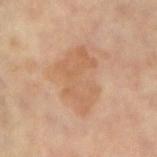The lesion was photographed on a routine skin check and not biopsied; there is no pathology result.
The total-body-photography lesion software estimated an eccentricity of roughly 0.8 and a symmetry-axis asymmetry near 0.3. It also reported radial color variation of about 1.
The patient is a male aged approximately 65.
Captured under cross-polarized illumination.
The lesion is located on the left arm.
Cropped from a total-body skin-imaging series; the visible field is about 15 mm.
Measured at roughly 7 mm in maximum diameter.